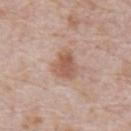Recorded during total-body skin imaging; not selected for excision or biopsy.
On the front of the torso.
A region of skin cropped from a whole-body photographic capture, roughly 15 mm wide.
A male subject, roughly 70 years of age.
Automated tile analysis of the lesion measured a border-irregularity index near 3/10, a within-lesion color-variation index near 3.5/10, and a peripheral color-asymmetry measure near 1. The software also gave a nevus-likeness score of about 70/100 and lesion-presence confidence of about 100/100.
The tile uses white-light illumination.